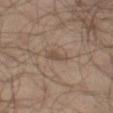– notes · no biopsy performed (imaged during a skin exam)
– automated lesion analysis · an eccentricity of roughly 0.9 and a shape-asymmetry score of about 0.4 (0 = symmetric)
– anatomic site · the leg
– image · ~15 mm tile from a whole-body skin photo
– patient · male, in their mid-60s
– size · about 3 mm